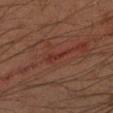Q: Who is the patient?
A: male, about 40 years old
Q: Lesion size?
A: about 3 mm
Q: What kind of image is this?
A: ~15 mm crop, total-body skin-cancer survey
Q: Where on the body is the lesion?
A: the left forearm